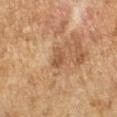The lesion is on the left forearm. Imaged with cross-polarized lighting. The lesion's longest dimension is about 2.5 mm. A 15 mm close-up tile from a total-body photography series done for melanoma screening. A female patient aged 58 to 62. Automated image analysis of the tile measured a footprint of about 3.5 mm² and two-axis asymmetry of about 0.3. The software also gave a lesion color around L≈46 a*≈18 b*≈30 in CIELAB, about 8 CIELAB-L* units darker than the surrounding skin, and a lesion-to-skin contrast of about 6.5 (normalized; higher = more distinct). The analysis additionally found a classifier nevus-likeness of about 0/100.